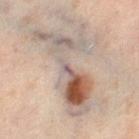Assessment: The lesion was photographed on a routine skin check and not biopsied; there is no pathology result. Clinical summary: Imaged with cross-polarized lighting. About 11 mm across. A female patient aged 48–52. Cropped from a total-body skin-imaging series; the visible field is about 15 mm. From the right thigh.